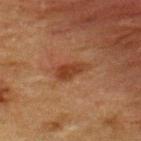biopsy status — total-body-photography surveillance lesion; no biopsy | lesion diameter — ~4 mm (longest diameter) | anatomic site — the upper back | automated lesion analysis — an area of roughly 6 mm², an eccentricity of roughly 0.9, and a shape-asymmetry score of about 0.35 (0 = symmetric); roughly 8 lightness units darker than nearby skin and a normalized lesion–skin contrast near 8; a nevus-likeness score of about 75/100 and a lesion-detection confidence of about 100/100 | imaging modality — total-body-photography crop, ~15 mm field of view | lighting — cross-polarized illumination | subject — male, aged around 50.